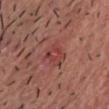<record>
<biopsy_status>not biopsied; imaged during a skin examination</biopsy_status>
<patient>
  <sex>male</sex>
  <age_approx>40</age_approx>
</patient>
<image>
  <source>total-body photography crop</source>
  <field_of_view_mm>15</field_of_view_mm>
</image>
<automated_metrics>
  <border_irregularity_0_10>4.0</border_irregularity_0_10>
  <color_variation_0_10>4.0</color_variation_0_10>
  <nevus_likeness_0_100>0</nevus_likeness_0_100>
  <lesion_detection_confidence_0_100>100</lesion_detection_confidence_0_100>
</automated_metrics>
<site>chest</site>
</record>